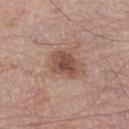follow-up: no biopsy performed (imaged during a skin exam) | patient: male, about 75 years old | location: the left thigh | automated lesion analysis: an area of roughly 8.5 mm², an eccentricity of roughly 0.6, and a symmetry-axis asymmetry near 0.2; an average lesion color of about L≈49 a*≈21 b*≈26 (CIELAB), roughly 11 lightness units darker than nearby skin, and a normalized border contrast of about 8.5; a classifier nevus-likeness of about 45/100 and lesion-presence confidence of about 100/100 | tile lighting: white-light illumination | image source: ~15 mm crop, total-body skin-cancer survey.